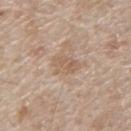Assessment:
Part of a total-body skin-imaging series; this lesion was reviewed on a skin check and was not flagged for biopsy.
Context:
A male patient, in their 80s. The lesion is on the mid back. A lesion tile, about 15 mm wide, cut from a 3D total-body photograph.